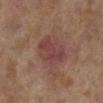Clinical impression:
The lesion was photographed on a routine skin check and not biopsied; there is no pathology result.
Clinical summary:
A female subject, aged 58–62. About 5 mm across. Located on the leg. Imaged with cross-polarized lighting. A 15 mm crop from a total-body photograph taken for skin-cancer surveillance.